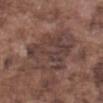| field | value |
|---|---|
| notes | no biopsy performed (imaged during a skin exam) |
| location | the abdomen |
| automated lesion analysis | a lesion color around L≈40 a*≈16 b*≈20 in CIELAB and about 7 CIELAB-L* units darker than the surrounding skin; a classifier nevus-likeness of about 0/100 and a detector confidence of about 70 out of 100 that the crop contains a lesion |
| lesion diameter | about 7 mm |
| imaging modality | total-body-photography crop, ~15 mm field of view |
| subject | male, approximately 75 years of age |